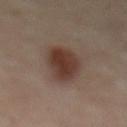No biopsy was performed on this lesion — it was imaged during a full skin examination and was not determined to be concerning.